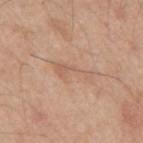The lesion was tiled from a total-body skin photograph and was not biopsied.
This image is a 15 mm lesion crop taken from a total-body photograph.
On the right upper arm.
The subject is a male approximately 55 years of age.
Approximately 4.5 mm at its widest.